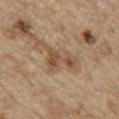The lesion was tiled from a total-body skin photograph and was not biopsied. A male subject, in their 70s. Automated image analysis of the tile measured an average lesion color of about L≈51 a*≈17 b*≈32 (CIELAB), a lesion–skin lightness drop of about 10, and a normalized border contrast of about 7. Approximately 5 mm at its widest. Captured under white-light illumination. A 15 mm close-up extracted from a 3D total-body photography capture. The lesion is on the front of the torso.